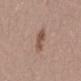follow-up: catalogued during a skin exam; not biopsied
patient: female, roughly 40 years of age
site: the lower back
lesion size: about 3 mm
image: ~15 mm crop, total-body skin-cancer survey
automated lesion analysis: a mean CIELAB color near L≈51 a*≈18 b*≈25, about 10 CIELAB-L* units darker than the surrounding skin, and a lesion-to-skin contrast of about 7 (normalized; higher = more distinct); an automated nevus-likeness rating near 50 out of 100 and lesion-presence confidence of about 100/100
lighting: white-light